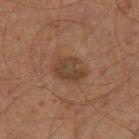Assessment:
Recorded during total-body skin imaging; not selected for excision or biopsy.
Background:
The tile uses cross-polarized illumination. A 15 mm crop from a total-body photograph taken for skin-cancer surveillance. The lesion is located on the leg. A male patient, roughly 60 years of age. An algorithmic analysis of the crop reported border irregularity of about 2 on a 0–10 scale and a within-lesion color-variation index near 3.5/10. Longest diameter approximately 4 mm.